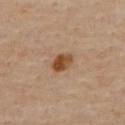No biopsy was performed on this lesion — it was imaged during a full skin examination and was not determined to be concerning. The lesion is on the upper back. The patient is a female roughly 60 years of age. Cropped from a whole-body photographic skin survey; the tile spans about 15 mm. Imaged with cross-polarized lighting. Longest diameter approximately 3 mm.